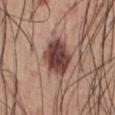Context:
About 4.5 mm across. A roughly 15 mm field-of-view crop from a total-body skin photograph. The subject is a male about 55 years old. The lesion is located on the abdomen. This is a white-light tile.